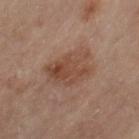biopsy status: total-body-photography surveillance lesion; no biopsy
body site: the left upper arm
lesion diameter: about 5.5 mm
acquisition: ~15 mm crop, total-body skin-cancer survey
patient: female, aged 63–67
image-analysis metrics: an area of roughly 16 mm², an outline eccentricity of about 0.7 (0 = round, 1 = elongated), and a symmetry-axis asymmetry near 0.25; a nevus-likeness score of about 25/100 and a lesion-detection confidence of about 100/100